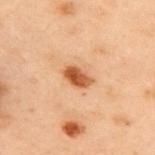• workup — total-body-photography surveillance lesion; no biopsy
• location — the upper back
• patient — male, approximately 55 years of age
• size — ~3 mm (longest diameter)
• image source — ~15 mm tile from a whole-body skin photo